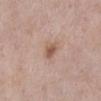notes: no biopsy performed (imaged during a skin exam) | subject: female, aged 63–67 | imaging modality: ~15 mm crop, total-body skin-cancer survey | image-analysis metrics: a lesion area of about 4 mm², a shape eccentricity near 0.6, and a symmetry-axis asymmetry near 0.25; an average lesion color of about L≈56 a*≈18 b*≈28 (CIELAB), roughly 10 lightness units darker than nearby skin, and a normalized border contrast of about 7; a border-irregularity rating of about 2/10, a color-variation rating of about 3/10, and radial color variation of about 1; a nevus-likeness score of about 50/100 and a detector confidence of about 100 out of 100 that the crop contains a lesion | lesion diameter: about 2.5 mm | body site: the left lower leg | lighting: white-light.